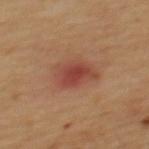| field | value |
|---|---|
| notes | no biopsy performed (imaged during a skin exam) |
| tile lighting | cross-polarized |
| body site | the back |
| patient | male, aged 58–62 |
| imaging modality | ~15 mm tile from a whole-body skin photo |